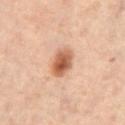follow-up: no biopsy performed (imaged during a skin exam); size: ≈3.5 mm; subject: male, aged around 60; image: ~15 mm crop, total-body skin-cancer survey; location: the abdomen.